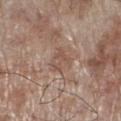Acquisition and patient details: The lesion is located on the left lower leg. A male patient about 70 years old. A roughly 15 mm field-of-view crop from a total-body skin photograph. Automated tile analysis of the lesion measured an area of roughly 5.5 mm², an outline eccentricity of about 0.25 (0 = round, 1 = elongated), and a shape-asymmetry score of about 0.4 (0 = symmetric). The analysis additionally found roughly 7 lightness units darker than nearby skin and a normalized lesion–skin contrast near 5. The analysis additionally found a border-irregularity index near 4/10 and radial color variation of about 1. The analysis additionally found a classifier nevus-likeness of about 0/100 and a detector confidence of about 85 out of 100 that the crop contains a lesion. Measured at roughly 3 mm in maximum diameter. Captured under white-light illumination.